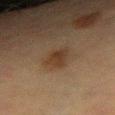The lesion was photographed on a routine skin check and not biopsied; there is no pathology result. A female patient, approximately 55 years of age. Located on the right forearm. Cropped from a total-body skin-imaging series; the visible field is about 15 mm.